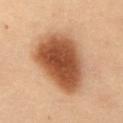Recorded during total-body skin imaging; not selected for excision or biopsy. On the front of the torso. A region of skin cropped from a whole-body photographic capture, roughly 15 mm wide. A male patient, approximately 55 years of age.